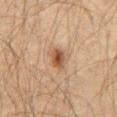follow-up: no biopsy performed (imaged during a skin exam)
image: 15 mm crop, total-body photography
patient: male, aged around 60
location: the abdomen